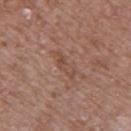Q: Was this lesion biopsied?
A: catalogued during a skin exam; not biopsied
Q: Patient demographics?
A: male, approximately 50 years of age
Q: How was the tile lit?
A: white-light illumination
Q: Automated lesion metrics?
A: an area of roughly 4.5 mm² and a shape eccentricity near 0.95; a lesion color around L≈48 a*≈20 b*≈27 in CIELAB and a normalized border contrast of about 5.5
Q: What is the anatomic site?
A: the back
Q: How was this image acquired?
A: ~15 mm tile from a whole-body skin photo